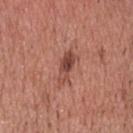biopsy status=imaged on a skin check; not biopsied | subject=male, aged 48 to 52 | site=the head or neck | image=~15 mm tile from a whole-body skin photo | lighting=white-light | lesion diameter=≈4 mm.